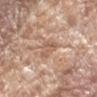tile lighting: white-light | lesion size: ~3 mm (longest diameter) | subject: male, aged 78 to 82 | imaging modality: ~15 mm tile from a whole-body skin photo | anatomic site: the arm.